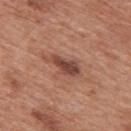This lesion was catalogued during total-body skin photography and was not selected for biopsy. The patient is a male about 70 years old. Longest diameter approximately 5 mm. A close-up tile cropped from a whole-body skin photograph, about 15 mm across. Imaged with white-light lighting. The lesion is on the mid back.